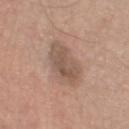Assessment:
Recorded during total-body skin imaging; not selected for excision or biopsy.
Background:
A 15 mm close-up extracted from a 3D total-body photography capture. The lesion is located on the left thigh. The total-body-photography lesion software estimated a detector confidence of about 100 out of 100 that the crop contains a lesion. Captured under white-light illumination. A male subject, aged 73–77. The lesion's longest dimension is about 5 mm.